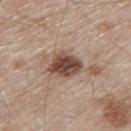The lesion was tiled from a total-body skin photograph and was not biopsied. A 15 mm crop from a total-body photograph taken for skin-cancer surveillance. A male patient, aged approximately 80. The lesion is on the mid back.